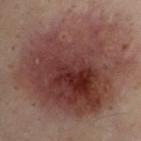{"biopsy_status": "not biopsied; imaged during a skin examination", "image": {"source": "total-body photography crop", "field_of_view_mm": 15}, "patient": {"sex": "male", "age_approx": 30}, "site": "front of the torso"}